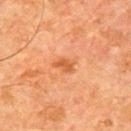{
  "biopsy_status": "not biopsied; imaged during a skin examination",
  "site": "arm",
  "lighting": "cross-polarized",
  "image": {
    "source": "total-body photography crop",
    "field_of_view_mm": 15
  },
  "lesion_size": {
    "long_diameter_mm_approx": 2.5
  },
  "patient": {
    "sex": "male",
    "age_approx": 65
  }
}